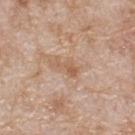No biopsy was performed on this lesion — it was imaged during a full skin examination and was not determined to be concerning. A 15 mm crop from a total-body photograph taken for skin-cancer surveillance. The total-body-photography lesion software estimated a classifier nevus-likeness of about 0/100 and a lesion-detection confidence of about 100/100. A male subject, aged around 80. Longest diameter approximately 3 mm. This is a white-light tile. The lesion is located on the upper back.